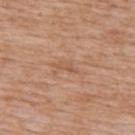Q: Was this lesion biopsied?
A: imaged on a skin check; not biopsied
Q: What kind of image is this?
A: ~15 mm crop, total-body skin-cancer survey
Q: What are the patient's age and sex?
A: male, roughly 60 years of age
Q: What is the anatomic site?
A: the upper back
Q: Lesion size?
A: ~2.5 mm (longest diameter)
Q: Automated lesion metrics?
A: a lesion color around L≈55 a*≈21 b*≈32 in CIELAB, roughly 7 lightness units darker than nearby skin, and a normalized border contrast of about 5; a border-irregularity index near 4.5/10 and peripheral color asymmetry of about 0; a classifier nevus-likeness of about 0/100 and a lesion-detection confidence of about 95/100